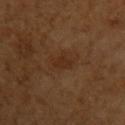follow-up: total-body-photography surveillance lesion; no biopsy | subject: female, aged around 55 | image source: ~15 mm crop, total-body skin-cancer survey | body site: the arm | diameter: about 3 mm.